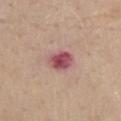Impression: No biopsy was performed on this lesion — it was imaged during a full skin examination and was not determined to be concerning. Acquisition and patient details: On the lower back. A female subject aged 63–67. A region of skin cropped from a whole-body photographic capture, roughly 15 mm wide. Captured under white-light illumination.